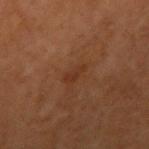Q: Is there a histopathology result?
A: no biopsy performed (imaged during a skin exam)
Q: How large is the lesion?
A: ~2.5 mm (longest diameter)
Q: What is the anatomic site?
A: the right upper arm
Q: Who is the patient?
A: male, aged 58–62
Q: What did automated image analysis measure?
A: an area of roughly 2.5 mm², an eccentricity of roughly 0.9, and a symmetry-axis asymmetry near 0.4; a classifier nevus-likeness of about 0/100 and lesion-presence confidence of about 100/100
Q: Illumination type?
A: cross-polarized illumination
Q: What is the imaging modality?
A: ~15 mm tile from a whole-body skin photo